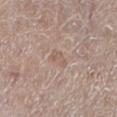Q: Is there a histopathology result?
A: no biopsy performed (imaged during a skin exam)
Q: Where on the body is the lesion?
A: the left lower leg
Q: Lesion size?
A: ≈2.5 mm
Q: Who is the patient?
A: female, roughly 50 years of age
Q: Automated lesion metrics?
A: an average lesion color of about L≈58 a*≈16 b*≈26 (CIELAB) and a normalized lesion–skin contrast near 5; a border-irregularity index near 3/10, internal color variation of about 1.5 on a 0–10 scale, and radial color variation of about 0.5; an automated nevus-likeness rating near 0 out of 100 and lesion-presence confidence of about 100/100
Q: Illumination type?
A: white-light illumination
Q: How was this image acquired?
A: 15 mm crop, total-body photography